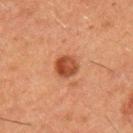Part of a total-body skin-imaging series; this lesion was reviewed on a skin check and was not flagged for biopsy. A male subject approximately 50 years of age. The tile uses cross-polarized illumination. Located on the left upper arm. A 15 mm close-up tile from a total-body photography series done for melanoma screening. Measured at roughly 3 mm in maximum diameter.